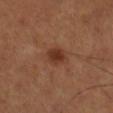Clinical summary:
The patient is a male roughly 50 years of age. Located on the right lower leg. Cropped from a whole-body photographic skin survey; the tile spans about 15 mm.